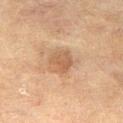No biopsy was performed on this lesion — it was imaged during a full skin examination and was not determined to be concerning. A male patient aged 68–72. The lesion is on the leg. A 15 mm close-up tile from a total-body photography series done for melanoma screening. The tile uses cross-polarized illumination.